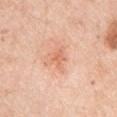Captured during whole-body skin photography for melanoma surveillance; the lesion was not biopsied.
The lesion is located on the arm.
A lesion tile, about 15 mm wide, cut from a 3D total-body photograph.
Automated tile analysis of the lesion measured a classifier nevus-likeness of about 5/100 and a detector confidence of about 100 out of 100 that the crop contains a lesion.
A male patient, approximately 70 years of age.
Measured at roughly 2.5 mm in maximum diameter.